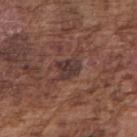Captured during whole-body skin photography for melanoma surveillance; the lesion was not biopsied. An algorithmic analysis of the crop reported a footprint of about 5 mm², an outline eccentricity of about 0.55 (0 = round, 1 = elongated), and a shape-asymmetry score of about 0.3 (0 = symmetric). It also reported a classifier nevus-likeness of about 0/100 and a detector confidence of about 80 out of 100 that the crop contains a lesion. Cropped from a total-body skin-imaging series; the visible field is about 15 mm. A male patient in their mid-70s. This is a white-light tile. The lesion is located on the right upper arm.